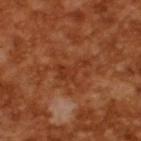This lesion was catalogued during total-body skin photography and was not selected for biopsy.
An algorithmic analysis of the crop reported a lesion area of about 5 mm² and two-axis asymmetry of about 0.6. And it measured an average lesion color of about L≈34 a*≈27 b*≈34 (CIELAB), about 6 CIELAB-L* units darker than the surrounding skin, and a lesion-to-skin contrast of about 5.5 (normalized; higher = more distinct).
A male subject, about 65 years old.
A lesion tile, about 15 mm wide, cut from a 3D total-body photograph.